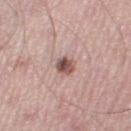This lesion was catalogued during total-body skin photography and was not selected for biopsy. On the left lower leg. Cropped from a total-body skin-imaging series; the visible field is about 15 mm. The tile uses white-light illumination. The subject is a male in their mid-50s. An algorithmic analysis of the crop reported a border-irregularity rating of about 1/10, a color-variation rating of about 6.5/10, and peripheral color asymmetry of about 2.5.